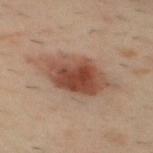- lesion diameter — ~6.5 mm (longest diameter)
- image — total-body-photography crop, ~15 mm field of view
- lighting — cross-polarized illumination
- subject — male, in their 40s
- body site — the upper back